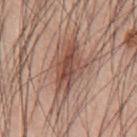Findings:
- workup — catalogued during a skin exam; not biopsied
- lighting — white-light illumination
- body site — the front of the torso
- patient — male, about 60 years old
- image source — ~15 mm tile from a whole-body skin photo
- lesion diameter — ≈6 mm